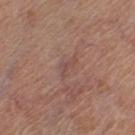Imaged during a routine full-body skin examination; the lesion was not biopsied and no histopathology is available. A female subject in their mid-50s. Measured at roughly 2.5 mm in maximum diameter. A 15 mm crop from a total-body photograph taken for skin-cancer surveillance. From the left thigh. Imaged with white-light lighting.